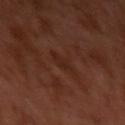follow-up: no biopsy performed (imaged during a skin exam); image source: 15 mm crop, total-body photography; anatomic site: the left upper arm; patient: male, in their 30s; lighting: cross-polarized illumination.